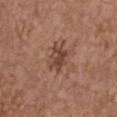The lesion was photographed on a routine skin check and not biopsied; there is no pathology result.
A lesion tile, about 15 mm wide, cut from a 3D total-body photograph.
A female patient, aged 63–67.
From the chest.